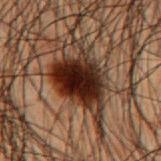biopsy status=catalogued during a skin exam; not biopsied
subject=male, aged 48 to 52
body site=the mid back
acquisition=~15 mm crop, total-body skin-cancer survey